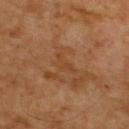Recorded during total-body skin imaging; not selected for excision or biopsy.
A 15 mm close-up tile from a total-body photography series done for melanoma screening.
The tile uses cross-polarized illumination.
On the back.
The subject is a male about 65 years old.
Longest diameter approximately 3 mm.
The total-body-photography lesion software estimated a mean CIELAB color near L≈41 a*≈21 b*≈35, roughly 5 lightness units darker than nearby skin, and a lesion-to-skin contrast of about 4.5 (normalized; higher = more distinct). And it measured a nevus-likeness score of about 0/100.